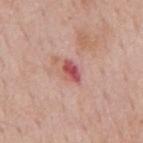Q: Was this lesion biopsied?
A: imaged on a skin check; not biopsied
Q: Lesion size?
A: ~3 mm (longest diameter)
Q: Where on the body is the lesion?
A: the mid back
Q: How was the tile lit?
A: white-light illumination
Q: Who is the patient?
A: male, aged 58–62
Q: What kind of image is this?
A: 15 mm crop, total-body photography